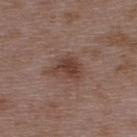The lesion was photographed on a routine skin check and not biopsied; there is no pathology result. The tile uses white-light illumination. The lesion is on the upper back. Longest diameter approximately 4 mm. This image is a 15 mm lesion crop taken from a total-body photograph. A male patient aged around 50.